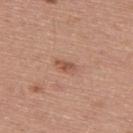* workup: no biopsy performed (imaged during a skin exam)